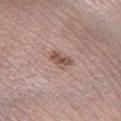Impression:
Imaged during a routine full-body skin examination; the lesion was not biopsied and no histopathology is available.
Background:
The patient is a male aged around 55. About 3 mm across. A roughly 15 mm field-of-view crop from a total-body skin photograph. This is a white-light tile. Automated image analysis of the tile measured a shape eccentricity near 0.8 and two-axis asymmetry of about 0.25. And it measured a border-irregularity index near 3/10, a within-lesion color-variation index near 3.5/10, and radial color variation of about 1.5. On the right lower leg.